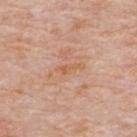Recorded during total-body skin imaging; not selected for excision or biopsy. Captured under white-light illumination. Measured at roughly 2.5 mm in maximum diameter. Cropped from a whole-body photographic skin survey; the tile spans about 15 mm. An algorithmic analysis of the crop reported a lesion color around L≈60 a*≈23 b*≈35 in CIELAB, roughly 6 lightness units darker than nearby skin, and a lesion-to-skin contrast of about 6 (normalized; higher = more distinct). It also reported a border-irregularity rating of about 4.5/10 and a within-lesion color-variation index near 0/10. It also reported an automated nevus-likeness rating near 0 out of 100 and lesion-presence confidence of about 100/100. The subject is a male aged 73–77. The lesion is located on the upper back.